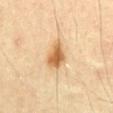This lesion was catalogued during total-body skin photography and was not selected for biopsy. A 15 mm close-up tile from a total-body photography series done for melanoma screening. Longest diameter approximately 3.5 mm. The subject is a male approximately 40 years of age. Located on the abdomen.